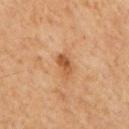<case>
<biopsy_status>not biopsied; imaged during a skin examination</biopsy_status>
<lighting>cross-polarized</lighting>
<lesion_size>
  <long_diameter_mm_approx>2.5</long_diameter_mm_approx>
</lesion_size>
<patient>
  <sex>male</sex>
  <age_approx>65</age_approx>
</patient>
<site>back</site>
<image>
  <source>total-body photography crop</source>
  <field_of_view_mm>15</field_of_view_mm>
</image>
</case>